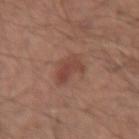The lesion was photographed on a routine skin check and not biopsied; there is no pathology result. A 15 mm close-up extracted from a 3D total-body photography capture. A male subject aged around 40. This is a white-light tile. The lesion is on the right forearm.